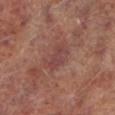Q: Was this lesion biopsied?
A: imaged on a skin check; not biopsied
Q: Patient demographics?
A: male, aged approximately 65
Q: What is the anatomic site?
A: the left lower leg
Q: How was this image acquired?
A: total-body-photography crop, ~15 mm field of view